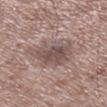follow-up — catalogued during a skin exam; not biopsied
TBP lesion metrics — an outline eccentricity of about 0.65 (0 = round, 1 = elongated) and a shape-asymmetry score of about 0.3 (0 = symmetric); a mean CIELAB color near L≈51 a*≈16 b*≈19, roughly 10 lightness units darker than nearby skin, and a lesion-to-skin contrast of about 7 (normalized; higher = more distinct); a border-irregularity index near 3.5/10, a within-lesion color-variation index near 4/10, and peripheral color asymmetry of about 1; a nevus-likeness score of about 0/100 and lesion-presence confidence of about 100/100
acquisition — ~15 mm crop, total-body skin-cancer survey
lighting — white-light
diameter — ≈5.5 mm
location — the right lower leg
subject — male, in their mid- to late 50s